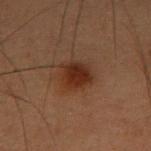The lesion was photographed on a routine skin check and not biopsied; there is no pathology result. Automated image analysis of the tile measured a lesion area of about 11 mm² and an eccentricity of roughly 0.35. The software also gave an average lesion color of about L≈25 a*≈18 b*≈24 (CIELAB) and about 8 CIELAB-L* units darker than the surrounding skin. It also reported a nevus-likeness score of about 95/100 and a detector confidence of about 100 out of 100 that the crop contains a lesion. A 15 mm close-up extracted from a 3D total-body photography capture. The lesion is on the right forearm. A male subject aged approximately 55. Approximately 4 mm at its widest. This is a cross-polarized tile.